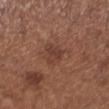| field | value |
|---|---|
| workup | total-body-photography surveillance lesion; no biopsy |
| automated metrics | a lesion area of about 4 mm² and an outline eccentricity of about 0.65 (0 = round, 1 = elongated); a lesion color around L≈39 a*≈21 b*≈26 in CIELAB, roughly 7 lightness units darker than nearby skin, and a normalized lesion–skin contrast near 6; a border-irregularity rating of about 4.5/10, a color-variation rating of about 1.5/10, and radial color variation of about 0.5; a classifier nevus-likeness of about 0/100 and lesion-presence confidence of about 100/100 |
| illumination | white-light |
| size | about 2.5 mm |
| anatomic site | the left lower leg |
| patient | female, approximately 55 years of age |
| image | ~15 mm crop, total-body skin-cancer survey |